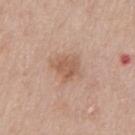Findings:
* follow-up: catalogued during a skin exam; not biopsied
* image source: ~15 mm crop, total-body skin-cancer survey
* illumination: white-light
* subject: male, in their 60s
* site: the chest
* automated metrics: an area of roughly 6.5 mm²; an average lesion color of about L≈57 a*≈20 b*≈30 (CIELAB), roughly 9 lightness units darker than nearby skin, and a lesion-to-skin contrast of about 6.5 (normalized; higher = more distinct); internal color variation of about 2 on a 0–10 scale